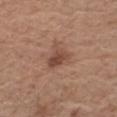Impression:
The lesion was photographed on a routine skin check and not biopsied; there is no pathology result.
Image and clinical context:
A male patient approximately 70 years of age. The lesion is located on the right upper arm. Cropped from a whole-body photographic skin survey; the tile spans about 15 mm.